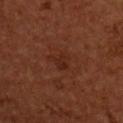| feature | finding |
|---|---|
| follow-up | total-body-photography surveillance lesion; no biopsy |
| image | total-body-photography crop, ~15 mm field of view |
| image-analysis metrics | a lesion area of about 4 mm²; an average lesion color of about L≈23 a*≈21 b*≈25 (CIELAB), roughly 4 lightness units darker than nearby skin, and a normalized border contrast of about 5.5; a border-irregularity index near 5.5/10 and internal color variation of about 2 on a 0–10 scale |
| tile lighting | cross-polarized |
| subject | male, about 55 years old |
| anatomic site | the upper back |